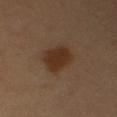Impression: This lesion was catalogued during total-body skin photography and was not selected for biopsy. Context: This is a cross-polarized tile. On the right upper arm. A 15 mm close-up tile from a total-body photography series done for melanoma screening. The lesion-visualizer software estimated about 9 CIELAB-L* units darker than the surrounding skin. And it measured a nevus-likeness score of about 100/100 and lesion-presence confidence of about 100/100. A male patient in their mid- to late 50s. The lesion's longest dimension is about 4 mm.